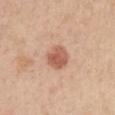biopsy_status: not biopsied; imaged during a skin examination
image:
  source: total-body photography crop
  field_of_view_mm: 15
lesion_size:
  long_diameter_mm_approx: 3.0
lighting: white-light
automated_metrics:
  vs_skin_darker_L: 12.0
  color_variation_0_10: 3.5
  peripheral_color_asymmetry: 1.0
  nevus_likeness_0_100: 100
  lesion_detection_confidence_0_100: 100
patient:
  sex: female
  age_approx: 45
site: chest